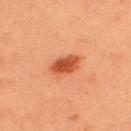{"biopsy_status": "not biopsied; imaged during a skin examination", "image": {"source": "total-body photography crop", "field_of_view_mm": 15}, "site": "upper back", "lesion_size": {"long_diameter_mm_approx": 3.5}, "automated_metrics": {"area_mm2_approx": 6.5, "eccentricity": 0.8, "shape_asymmetry": 0.2, "cielab_L": 52, "cielab_a": 33, "cielab_b": 41, "vs_skin_contrast_norm": 9.5, "border_irregularity_0_10": 2.0, "color_variation_0_10": 3.5, "peripheral_color_asymmetry": 1.0, "nevus_likeness_0_100": 100, "lesion_detection_confidence_0_100": 100}, "patient": {"sex": "male", "age_approx": 35}, "lighting": "cross-polarized"}